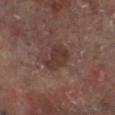biopsy status=total-body-photography surveillance lesion; no biopsy
diameter=≈3.5 mm
subject=male, approximately 65 years of age
anatomic site=the left lower leg
acquisition=~15 mm tile from a whole-body skin photo
lighting=cross-polarized